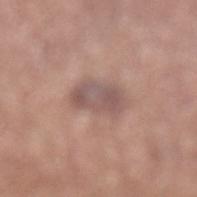follow-up: total-body-photography surveillance lesion; no biopsy | body site: the left lower leg | TBP lesion metrics: an average lesion color of about L≈54 a*≈18 b*≈21 (CIELAB), a lesion–skin lightness drop of about 9, and a lesion-to-skin contrast of about 7 (normalized; higher = more distinct); border irregularity of about 2.5 on a 0–10 scale, internal color variation of about 4 on a 0–10 scale, and a peripheral color-asymmetry measure near 1; a nevus-likeness score of about 0/100 and a lesion-detection confidence of about 100/100 | diameter: ≈4 mm | subject: male, aged 68 to 72 | image source: 15 mm crop, total-body photography | tile lighting: white-light illumination.